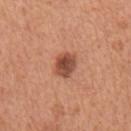<tbp_lesion>
<biopsy_status>not biopsied; imaged during a skin examination</biopsy_status>
<image>
  <source>total-body photography crop</source>
  <field_of_view_mm>15</field_of_view_mm>
</image>
<site>back</site>
<automated_metrics>
  <nevus_likeness_0_100>85</nevus_likeness_0_100>
</automated_metrics>
<lighting>white-light</lighting>
<lesion_size>
  <long_diameter_mm_approx>3.0</long_diameter_mm_approx>
</lesion_size>
<patient>
  <sex>male</sex>
  <age_approx>65</age_approx>
</patient>
</tbp_lesion>